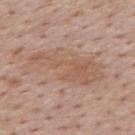  biopsy_status: not biopsied; imaged during a skin examination
  site: back
  patient:
    sex: male
    age_approx: 75
  image:
    source: total-body photography crop
    field_of_view_mm: 15
  lighting: white-light